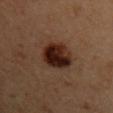The lesion was tiled from a total-body skin photograph and was not biopsied. The lesion is located on the left upper arm. The tile uses cross-polarized illumination. The subject is a male aged 33 to 37. About 4.5 mm across. A close-up tile cropped from a whole-body skin photograph, about 15 mm across.